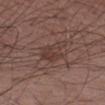A male subject aged 63 to 67. Located on the arm. A region of skin cropped from a whole-body photographic capture, roughly 15 mm wide. The tile uses white-light illumination.